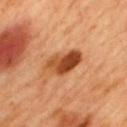follow-up=imaged on a skin check; not biopsied | tile lighting=cross-polarized | imaging modality=15 mm crop, total-body photography | body site=the back | lesion diameter=~5.5 mm (longest diameter) | patient=male, aged 48 to 52.